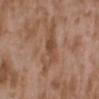Captured during whole-body skin photography for melanoma surveillance; the lesion was not biopsied.
On the upper back.
Cropped from a total-body skin-imaging series; the visible field is about 15 mm.
A female patient aged 33–37.
Longest diameter approximately 7 mm.
This is a white-light tile.